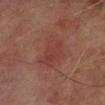Context: A roughly 15 mm field-of-view crop from a total-body skin photograph. A male patient roughly 70 years of age. About 5 mm across. The tile uses cross-polarized illumination. Automated image analysis of the tile measured a lesion area of about 12 mm² and an eccentricity of roughly 0.7. The analysis additionally found border irregularity of about 3.5 on a 0–10 scale, a color-variation rating of about 2/10, and a peripheral color-asymmetry measure near 0.5. The software also gave a nevus-likeness score of about 5/100. Located on the left lower leg.